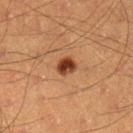notes = total-body-photography surveillance lesion; no biopsy | tile lighting = cross-polarized | acquisition = ~15 mm crop, total-body skin-cancer survey | diameter = ~2.5 mm (longest diameter) | site = the leg | patient = male, approximately 40 years of age.